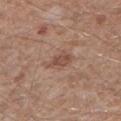follow-up: total-body-photography surveillance lesion; no biopsy | patient: male, aged approximately 65 | TBP lesion metrics: a footprint of about 5 mm², a shape eccentricity near 0.8, and a symmetry-axis asymmetry near 0.3; a mean CIELAB color near L≈49 a*≈20 b*≈26 and a lesion–skin lightness drop of about 9; an automated nevus-likeness rating near 5 out of 100 and lesion-presence confidence of about 100/100 | acquisition: ~15 mm crop, total-body skin-cancer survey | size: ~3 mm (longest diameter) | site: the left lower leg.